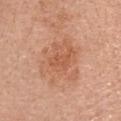Assessment: Part of a total-body skin-imaging series; this lesion was reviewed on a skin check and was not flagged for biopsy. Acquisition and patient details: A 15 mm close-up extracted from a 3D total-body photography capture. The patient is a female in their mid- to late 60s. The lesion is on the head or neck. Approximately 3 mm at its widest. Captured under white-light illumination. The lesion-visualizer software estimated a footprint of about 3.5 mm², an outline eccentricity of about 0.8 (0 = round, 1 = elongated), and a shape-asymmetry score of about 0.3 (0 = symmetric). It also reported about 7 CIELAB-L* units darker than the surrounding skin. The software also gave internal color variation of about 1.5 on a 0–10 scale.